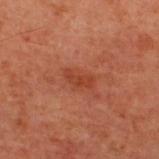site = the upper back | illumination = cross-polarized | subject = male, aged around 60 | imaging modality = total-body-photography crop, ~15 mm field of view | lesion diameter = ≈3 mm.